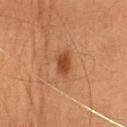Imaged during a routine full-body skin examination; the lesion was not biopsied and no histopathology is available. From the head or neck. The recorded lesion diameter is about 2.5 mm. Automated tile analysis of the lesion measured a lesion color around L≈37 a*≈22 b*≈32 in CIELAB, a lesion–skin lightness drop of about 9, and a normalized lesion–skin contrast near 8.5. The analysis additionally found a border-irregularity rating of about 2.5/10 and a peripheral color-asymmetry measure near 0.5. The tile uses cross-polarized illumination. A lesion tile, about 15 mm wide, cut from a 3D total-body photograph. The subject is a female approximately 60 years of age.